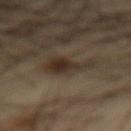The patient is a male about 60 years old. A lesion tile, about 15 mm wide, cut from a 3D total-body photograph. The recorded lesion diameter is about 5 mm. Automated tile analysis of the lesion measured a border-irregularity index near 5/10, a color-variation rating of about 5/10, and radial color variation of about 1.5. And it measured a nevus-likeness score of about 85/100 and a detector confidence of about 95 out of 100 that the crop contains a lesion. The lesion is located on the abdomen.